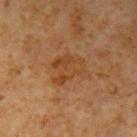No biopsy was performed on this lesion — it was imaged during a full skin examination and was not determined to be concerning.